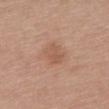{"biopsy_status": "not biopsied; imaged during a skin examination"}